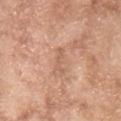{"biopsy_status": "not biopsied; imaged during a skin examination", "image": {"source": "total-body photography crop", "field_of_view_mm": 15}, "lighting": "white-light", "patient": {"sex": "male", "age_approx": 65}, "site": "front of the torso"}